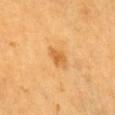This lesion was catalogued during total-body skin photography and was not selected for biopsy.
Located on the chest.
A lesion tile, about 15 mm wide, cut from a 3D total-body photograph.
The total-body-photography lesion software estimated a lesion area of about 3.5 mm², a shape eccentricity near 0.75, and a symmetry-axis asymmetry near 0.2. The software also gave an average lesion color of about L≈62 a*≈25 b*≈49 (CIELAB) and a lesion-to-skin contrast of about 6.5 (normalized; higher = more distinct). The analysis additionally found a border-irregularity rating of about 2/10 and peripheral color asymmetry of about 0.5. The software also gave an automated nevus-likeness rating near 80 out of 100 and a lesion-detection confidence of about 100/100.
The tile uses cross-polarized illumination.
A male patient, approximately 60 years of age.
Approximately 2.5 mm at its widest.